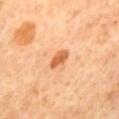Impression:
Recorded during total-body skin imaging; not selected for excision or biopsy.
Image and clinical context:
A 15 mm close-up extracted from a 3D total-body photography capture. Imaged with cross-polarized lighting. The lesion is on the mid back. A female patient, about 65 years old. Automated image analysis of the tile measured about 13 CIELAB-L* units darker than the surrounding skin and a normalized lesion–skin contrast near 8.5. It also reported border irregularity of about 3 on a 0–10 scale, internal color variation of about 2 on a 0–10 scale, and a peripheral color-asymmetry measure near 0.5. And it measured a classifier nevus-likeness of about 90/100. Approximately 3 mm at its widest.